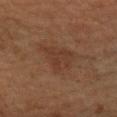Longest diameter approximately 5 mm.
From the right forearm.
The patient is a female aged 38 to 42.
Cropped from a whole-body photographic skin survey; the tile spans about 15 mm.
Captured under cross-polarized illumination.